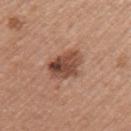<tbp_lesion>
<biopsy_status>not biopsied; imaged during a skin examination</biopsy_status>
<site>left upper arm</site>
<lesion_size>
  <long_diameter_mm_approx>5.0</long_diameter_mm_approx>
</lesion_size>
<automated_metrics>
  <area_mm2_approx>11.0</area_mm2_approx>
  <eccentricity>0.8</eccentricity>
  <shape_asymmetry>0.2</shape_asymmetry>
  <cielab_L>47</cielab_L>
  <cielab_a>22</cielab_a>
  <cielab_b>29</cielab_b>
  <vs_skin_darker_L>13.0</vs_skin_darker_L>
  <vs_skin_contrast_norm>9.5</vs_skin_contrast_norm>
  <nevus_likeness_0_100>80</nevus_likeness_0_100>
  <lesion_detection_confidence_0_100>100</lesion_detection_confidence_0_100>
</automated_metrics>
<patient>
  <sex>female</sex>
  <age_approx>50</age_approx>
</patient>
<image>
  <source>total-body photography crop</source>
  <field_of_view_mm>15</field_of_view_mm>
</image>
</tbp_lesion>